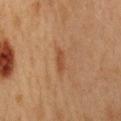Q: Is there a histopathology result?
A: imaged on a skin check; not biopsied
Q: Where on the body is the lesion?
A: the chest
Q: How was this image acquired?
A: total-body-photography crop, ~15 mm field of view
Q: Lesion size?
A: about 3 mm
Q: What did automated image analysis measure?
A: a lesion area of about 2.5 mm², an outline eccentricity of about 0.95 (0 = round, 1 = elongated), and a shape-asymmetry score of about 0.35 (0 = symmetric); a border-irregularity index near 3.5/10; an automated nevus-likeness rating near 45 out of 100 and a lesion-detection confidence of about 100/100
Q: Illumination type?
A: cross-polarized
Q: Who is the patient?
A: female, aged approximately 40